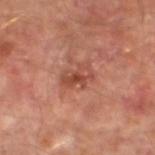Q: Was this lesion biopsied?
A: total-body-photography surveillance lesion; no biopsy
Q: Where on the body is the lesion?
A: the leg
Q: What did automated image analysis measure?
A: lesion-presence confidence of about 100/100
Q: How was this image acquired?
A: ~15 mm tile from a whole-body skin photo
Q: How was the tile lit?
A: cross-polarized
Q: Who is the patient?
A: male, aged around 65
Q: How large is the lesion?
A: about 3.5 mm